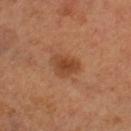workup: imaged on a skin check; not biopsied | patient: female, about 60 years old | size: ≈3.5 mm | location: the left lower leg | imaging modality: ~15 mm crop, total-body skin-cancer survey | automated metrics: about 10 CIELAB-L* units darker than the surrounding skin and a lesion-to-skin contrast of about 7.5 (normalized; higher = more distinct); a border-irregularity index near 3/10 and a color-variation rating of about 3/10; an automated nevus-likeness rating near 70 out of 100 and a detector confidence of about 100 out of 100 that the crop contains a lesion.